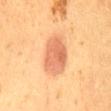Q: Is there a histopathology result?
A: no biopsy performed (imaged during a skin exam)
Q: Where on the body is the lesion?
A: the lower back
Q: What is the imaging modality?
A: ~15 mm crop, total-body skin-cancer survey
Q: Patient demographics?
A: female, about 50 years old